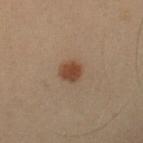notes: total-body-photography surveillance lesion; no biopsy
subject: male, approximately 55 years of age
location: the left forearm
image source: total-body-photography crop, ~15 mm field of view
illumination: cross-polarized
size: about 2.5 mm